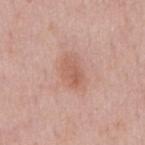Impression: No biopsy was performed on this lesion — it was imaged during a full skin examination and was not determined to be concerning. Context: An algorithmic analysis of the crop reported a border-irregularity index near 2.5/10 and a color-variation rating of about 3.5/10. And it measured a lesion-detection confidence of about 100/100. A roughly 15 mm field-of-view crop from a total-body skin photograph. The patient is a male roughly 55 years of age. On the mid back.